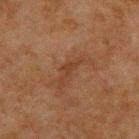biopsy status: total-body-photography surveillance lesion; no biopsy | location: the upper back | imaging modality: ~15 mm tile from a whole-body skin photo | patient: male, aged 58 to 62 | lighting: cross-polarized illumination | size: ≈4 mm.